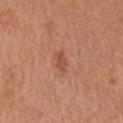Case summary:
– biopsy status · total-body-photography surveillance lesion; no biopsy
– image · 15 mm crop, total-body photography
– location · the left upper arm
– size · ≈3 mm
– tile lighting · white-light illumination
– subject · male, roughly 65 years of age